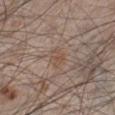{
  "biopsy_status": "not biopsied; imaged during a skin examination",
  "site": "left lower leg",
  "patient": {
    "sex": "male",
    "age_approx": 60
  },
  "automated_metrics": {
    "area_mm2_approx": 4.5,
    "eccentricity": 0.55,
    "shape_asymmetry": 0.2,
    "nevus_likeness_0_100": 0
  },
  "image": {
    "source": "total-body photography crop",
    "field_of_view_mm": 15
  },
  "lighting": "white-light"
}